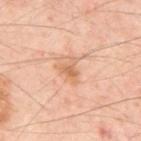Background: This image is a 15 mm lesion crop taken from a total-body photograph. On the back. Longest diameter approximately 3 mm. The total-body-photography lesion software estimated a footprint of about 4.5 mm², an outline eccentricity of about 0.6 (0 = round, 1 = elongated), and a symmetry-axis asymmetry near 0.5. It also reported about 9 CIELAB-L* units darker than the surrounding skin and a normalized lesion–skin contrast near 6. And it measured a nevus-likeness score of about 0/100 and a lesion-detection confidence of about 100/100. The patient is about 55 years old. This is a cross-polarized tile.